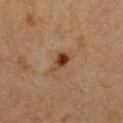Imaged during a routine full-body skin examination; the lesion was not biopsied and no histopathology is available. From the front of the torso. This image is a 15 mm lesion crop taken from a total-body photograph. A male subject, roughly 65 years of age.